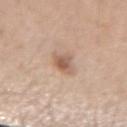Part of a total-body skin-imaging series; this lesion was reviewed on a skin check and was not flagged for biopsy. Approximately 3 mm at its widest. The subject is a female aged 43–47. A lesion tile, about 15 mm wide, cut from a 3D total-body photograph. The lesion-visualizer software estimated a border-irregularity rating of about 2.5/10, a color-variation rating of about 4.5/10, and peripheral color asymmetry of about 1.5. And it measured a lesion-detection confidence of about 100/100. The lesion is on the arm.